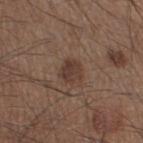follow-up=no biopsy performed (imaged during a skin exam)
body site=the right thigh
subject=male, roughly 45 years of age
image-analysis metrics=border irregularity of about 1.5 on a 0–10 scale, a color-variation rating of about 3/10, and peripheral color asymmetry of about 1; a nevus-likeness score of about 55/100
image=15 mm crop, total-body photography
tile lighting=white-light illumination